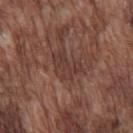The lesion was tiled from a total-body skin photograph and was not biopsied. This image is a 15 mm lesion crop taken from a total-body photograph. The subject is a male aged 73–77. Imaged with white-light lighting. About 3.5 mm across. On the chest.